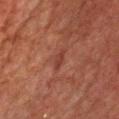This is a cross-polarized tile.
A 15 mm close-up tile from a total-body photography series done for melanoma screening.
Automated tile analysis of the lesion measured an automated nevus-likeness rating near 0 out of 100 and a lesion-detection confidence of about 80/100.
On the front of the torso.
The subject is a male aged approximately 60.